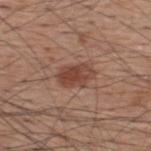biopsy_status: not biopsied; imaged during a skin examination
lighting: white-light
patient:
  sex: male
  age_approx: 60
site: back
image:
  source: total-body photography crop
  field_of_view_mm: 15
automated_metrics:
  area_mm2_approx: 8.0
  eccentricity: 0.8
  shape_asymmetry: 0.2
  cielab_L: 44
  cielab_a: 22
  cielab_b: 28
  vs_skin_darker_L: 10.0
  vs_skin_contrast_norm: 8.0
  nevus_likeness_0_100: 90
  lesion_detection_confidence_0_100: 100
lesion_size:
  long_diameter_mm_approx: 4.0